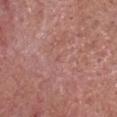* follow-up — imaged on a skin check; not biopsied
* size — about 1 mm
* lighting — white-light
* acquisition — ~15 mm tile from a whole-body skin photo
* patient — male, aged 78–82
* body site — the head or neck
* automated metrics — a border-irregularity index near 2.5/10, internal color variation of about 0 on a 0–10 scale, and a peripheral color-asymmetry measure near 0